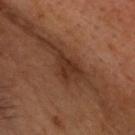Notes:
– workup: no biopsy performed (imaged during a skin exam)
– tile lighting: cross-polarized
– imaging modality: total-body-photography crop, ~15 mm field of view
– body site: the head or neck
– subject: male, aged 53 to 57
– lesion size: ~3 mm (longest diameter)